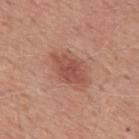Context: Measured at roughly 5 mm in maximum diameter. Located on the mid back. This image is a 15 mm lesion crop taken from a total-body photograph. The tile uses white-light illumination. An algorithmic analysis of the crop reported roughly 10 lightness units darker than nearby skin. The analysis additionally found a border-irregularity index near 3/10, a within-lesion color-variation index near 2.5/10, and a peripheral color-asymmetry measure near 1. A male patient, in their 50s.